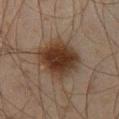Part of a total-body skin-imaging series; this lesion was reviewed on a skin check and was not flagged for biopsy. The recorded lesion diameter is about 4.5 mm. Located on the right thigh. Automated tile analysis of the lesion measured an average lesion color of about L≈27 a*≈14 b*≈23 (CIELAB), a lesion–skin lightness drop of about 12, and a normalized lesion–skin contrast near 12. A male patient in their mid- to late 40s. This is a cross-polarized tile. A roughly 15 mm field-of-view crop from a total-body skin photograph.